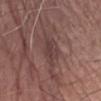{
  "biopsy_status": "not biopsied; imaged during a skin examination",
  "site": "right forearm",
  "patient": {
    "sex": "male",
    "age_approx": 80
  },
  "image": {
    "source": "total-body photography crop",
    "field_of_view_mm": 15
  },
  "lighting": "white-light",
  "lesion_size": {
    "long_diameter_mm_approx": 4.0
  }
}